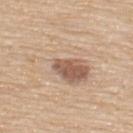This lesion was catalogued during total-body skin photography and was not selected for biopsy. Imaged with white-light lighting. Longest diameter approximately 8.5 mm. A 15 mm crop from a total-body photograph taken for skin-cancer surveillance. On the upper back. A male subject about 80 years old. Automated image analysis of the tile measured a nevus-likeness score of about 75/100 and a detector confidence of about 100 out of 100 that the crop contains a lesion.